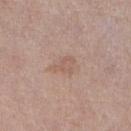Q: Was this lesion biopsied?
A: no biopsy performed (imaged during a skin exam)
Q: How large is the lesion?
A: ≈2.5 mm
Q: What did automated image analysis measure?
A: an area of roughly 4 mm², a shape eccentricity near 0.65, and two-axis asymmetry of about 0.45; a border-irregularity index near 4.5/10 and a color-variation rating of about 2/10
Q: Who is the patient?
A: male, aged approximately 70
Q: What is the imaging modality?
A: ~15 mm tile from a whole-body skin photo
Q: What is the anatomic site?
A: the right lower leg